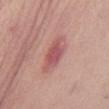biopsy status = catalogued during a skin exam; not biopsied | lighting = white-light illumination | subject = female, in their mid- to late 60s | anatomic site = the abdomen | lesion size = ~4.5 mm (longest diameter) | image source = total-body-photography crop, ~15 mm field of view.